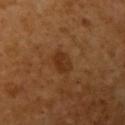Clinical impression:
Imaged during a routine full-body skin examination; the lesion was not biopsied and no histopathology is available.
Context:
The recorded lesion diameter is about 3 mm. A close-up tile cropped from a whole-body skin photograph, about 15 mm across. Automated image analysis of the tile measured a border-irregularity index near 1.5/10, internal color variation of about 2.5 on a 0–10 scale, and radial color variation of about 1. This is a cross-polarized tile. On the left upper arm. The patient is a female approximately 55 years of age.